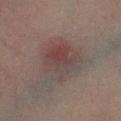Clinical impression:
Imaged during a routine full-body skin examination; the lesion was not biopsied and no histopathology is available.
Acquisition and patient details:
A male patient aged approximately 75. A 15 mm close-up extracted from a 3D total-body photography capture. Measured at roughly 5 mm in maximum diameter. From the left lower leg. Captured under cross-polarized illumination.